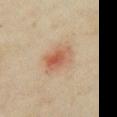patient:
  sex: female
  age_approx: 35
site: chest
lesion_size:
  long_diameter_mm_approx: 4.0
lighting: cross-polarized
image:
  source: total-body photography crop
  field_of_view_mm: 15